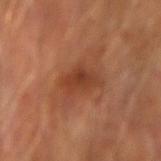Case summary:
– body site: the left forearm
– size: about 5 mm
– imaging modality: ~15 mm crop, total-body skin-cancer survey
– tile lighting: cross-polarized illumination
– patient: male, in their mid- to late 60s